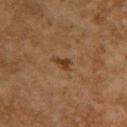| feature | finding |
|---|---|
| follow-up | total-body-photography surveillance lesion; no biopsy |
| lighting | cross-polarized illumination |
| diameter | about 2.5 mm |
| subject | female, aged 58–62 |
| image source | ~15 mm tile from a whole-body skin photo |
| site | the upper back |